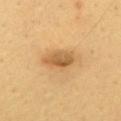Case summary:
• workup · no biopsy performed (imaged during a skin exam)
• image source · ~15 mm crop, total-body skin-cancer survey
• body site · the chest
• tile lighting · cross-polarized illumination
• subject · male, aged approximately 65